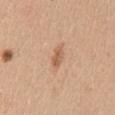Background:
The patient is a female aged approximately 30. The lesion is on the chest. Cropped from a whole-body photographic skin survey; the tile spans about 15 mm.